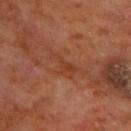This lesion was catalogued during total-body skin photography and was not selected for biopsy. Located on the chest. The total-body-photography lesion software estimated an average lesion color of about L≈39 a*≈24 b*≈31 (CIELAB), a lesion–skin lightness drop of about 6, and a lesion-to-skin contrast of about 5.5 (normalized; higher = more distinct). And it measured a border-irregularity rating of about 9/10 and a peripheral color-asymmetry measure near 0.5. The software also gave an automated nevus-likeness rating near 0 out of 100 and a lesion-detection confidence of about 75/100. A roughly 15 mm field-of-view crop from a total-body skin photograph. This is a cross-polarized tile. A male subject aged 58 to 62. The recorded lesion diameter is about 5.5 mm.